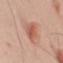| field | value |
|---|---|
| notes | catalogued during a skin exam; not biopsied |
| automated lesion analysis | a footprint of about 10 mm² and two-axis asymmetry of about 0.25; roughly 10 lightness units darker than nearby skin and a lesion-to-skin contrast of about 7 (normalized; higher = more distinct); a nevus-likeness score of about 100/100 and a detector confidence of about 100 out of 100 that the crop contains a lesion |
| patient | male, aged 48 to 52 |
| anatomic site | the left upper arm |
| tile lighting | white-light |
| imaging modality | ~15 mm tile from a whole-body skin photo |
| lesion size | ~6 mm (longest diameter) |